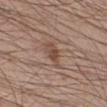Impression: This lesion was catalogued during total-body skin photography and was not selected for biopsy. Context: The lesion's longest dimension is about 3 mm. A male subject, aged 33 to 37. From the right lower leg. This image is a 15 mm lesion crop taken from a total-body photograph. An algorithmic analysis of the crop reported a shape eccentricity near 0.85 and two-axis asymmetry of about 0.3. It also reported a nevus-likeness score of about 50/100 and a lesion-detection confidence of about 100/100. This is a white-light tile.